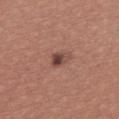Imaged during a routine full-body skin examination; the lesion was not biopsied and no histopathology is available.
A 15 mm crop from a total-body photograph taken for skin-cancer surveillance.
A female subject aged approximately 20.
The tile uses white-light illumination.
Measured at roughly 2.5 mm in maximum diameter.
The lesion is located on the upper back.